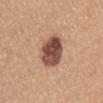Q: Is there a histopathology result?
A: total-body-photography surveillance lesion; no biopsy
Q: What kind of image is this?
A: ~15 mm tile from a whole-body skin photo
Q: What is the lesion's diameter?
A: ≈4.5 mm
Q: What are the patient's age and sex?
A: female, aged 53 to 57
Q: What is the anatomic site?
A: the abdomen
Q: Illumination type?
A: white-light illumination
Q: What did automated image analysis measure?
A: a lesion area of about 13 mm² and a symmetry-axis asymmetry near 0.2; an average lesion color of about L≈49 a*≈22 b*≈27 (CIELAB), a lesion–skin lightness drop of about 18, and a normalized lesion–skin contrast near 12.5; border irregularity of about 1.5 on a 0–10 scale, a within-lesion color-variation index near 5.5/10, and a peripheral color-asymmetry measure near 2